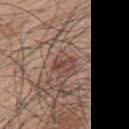No biopsy was performed on this lesion — it was imaged during a full skin examination and was not determined to be concerning.
Longest diameter approximately 2.5 mm.
A region of skin cropped from a whole-body photographic capture, roughly 15 mm wide.
A male subject roughly 70 years of age.
Located on the arm.
Imaged with white-light lighting.